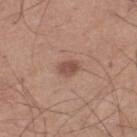Impression: Part of a total-body skin-imaging series; this lesion was reviewed on a skin check and was not flagged for biopsy. Background: About 2.5 mm across. Captured under white-light illumination. A roughly 15 mm field-of-view crop from a total-body skin photograph. Located on the right lower leg. The subject is a male roughly 60 years of age. Automated tile analysis of the lesion measured a border-irregularity index near 1/10, a color-variation rating of about 2/10, and peripheral color asymmetry of about 1. And it measured a classifier nevus-likeness of about 70/100 and a detector confidence of about 100 out of 100 that the crop contains a lesion.